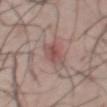Assessment: No biopsy was performed on this lesion — it was imaged during a full skin examination and was not determined to be concerning. Context: Approximately 4 mm at its widest. A male patient in their mid- to late 50s. This is a white-light tile. The lesion is on the chest. The lesion-visualizer software estimated an average lesion color of about L≈50 a*≈22 b*≈21 (CIELAB), a lesion–skin lightness drop of about 9, and a normalized lesion–skin contrast near 7. And it measured a border-irregularity rating of about 4/10, a within-lesion color-variation index near 1.5/10, and a peripheral color-asymmetry measure near 0.5. It also reported an automated nevus-likeness rating near 5 out of 100 and lesion-presence confidence of about 75/100. Cropped from a whole-body photographic skin survey; the tile spans about 15 mm.